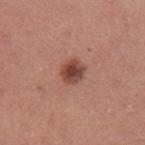workup=total-body-photography surveillance lesion; no biopsy
image=15 mm crop, total-body photography
diameter=about 3 mm
lighting=white-light
anatomic site=the left upper arm
patient=female, aged 23–27